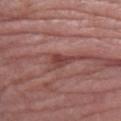<record>
<biopsy_status>not biopsied; imaged during a skin examination</biopsy_status>
<lighting>white-light</lighting>
<image>
  <source>total-body photography crop</source>
  <field_of_view_mm>15</field_of_view_mm>
</image>
<lesion_size>
  <long_diameter_mm_approx>3.0</long_diameter_mm_approx>
</lesion_size>
<patient>
  <sex>female</sex>
  <age_approx>65</age_approx>
</patient>
<automated_metrics>
  <area_mm2_approx>3.5</area_mm2_approx>
  <eccentricity>0.85</eccentricity>
  <vs_skin_darker_L>10.0</vs_skin_darker_L>
  <vs_skin_contrast_norm>8.0</vs_skin_contrast_norm>
  <lesion_detection_confidence_0_100>75</lesion_detection_confidence_0_100>
</automated_metrics>
<site>arm</site>
</record>